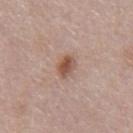Findings:
* notes · total-body-photography surveillance lesion; no biopsy
* image-analysis metrics · an area of roughly 4.5 mm² and an outline eccentricity of about 0.6 (0 = round, 1 = elongated); a lesion color around L≈52 a*≈20 b*≈27 in CIELAB, about 11 CIELAB-L* units darker than the surrounding skin, and a lesion-to-skin contrast of about 8.5 (normalized; higher = more distinct); a nevus-likeness score of about 90/100 and a detector confidence of about 100 out of 100 that the crop contains a lesion
* image · ~15 mm tile from a whole-body skin photo
* subject · male, aged approximately 55
* lesion size · about 2.5 mm
* tile lighting · white-light
* site · the front of the torso